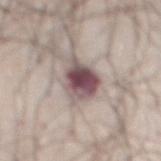biopsy_status: not biopsied; imaged during a skin examination
image:
  source: total-body photography crop
  field_of_view_mm: 15
patient:
  sex: male
  age_approx: 70
site: abdomen
lighting: white-light
lesion_size:
  long_diameter_mm_approx: 5.5